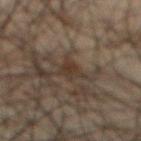Findings:
• follow-up: catalogued during a skin exam; not biopsied
• subject: male, about 65 years old
• body site: the abdomen
• image: 15 mm crop, total-body photography
• illumination: cross-polarized illumination
• lesion diameter: about 3 mm